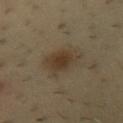follow-up: catalogued during a skin exam; not biopsied | image: ~15 mm crop, total-body skin-cancer survey | size: ~4.5 mm (longest diameter) | automated metrics: an average lesion color of about L≈38 a*≈11 b*≈27 (CIELAB) and roughly 8 lightness units darker than nearby skin; border irregularity of about 3 on a 0–10 scale, internal color variation of about 3.5 on a 0–10 scale, and radial color variation of about 1; a nevus-likeness score of about 85/100 and lesion-presence confidence of about 100/100 | patient: female, about 40 years old | site: the chest.